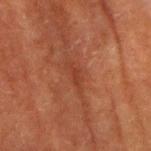<record>
<biopsy_status>not biopsied; imaged during a skin examination</biopsy_status>
<site>right upper arm</site>
<image>
  <source>total-body photography crop</source>
  <field_of_view_mm>15</field_of_view_mm>
</image>
<lighting>cross-polarized</lighting>
<patient>
  <sex>male</sex>
  <age_approx>75</age_approx>
</patient>
</record>